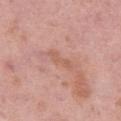Q: Was this lesion biopsied?
A: total-body-photography surveillance lesion; no biopsy
Q: Automated lesion metrics?
A: a mean CIELAB color near L≈59 a*≈23 b*≈29, about 6 CIELAB-L* units darker than the surrounding skin, and a normalized border contrast of about 5; a nevus-likeness score of about 0/100
Q: What kind of image is this?
A: total-body-photography crop, ~15 mm field of view
Q: What is the anatomic site?
A: the left thigh
Q: Patient demographics?
A: female, aged 48–52
Q: Illumination type?
A: white-light illumination